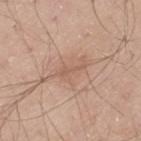Assessment: Recorded during total-body skin imaging; not selected for excision or biopsy. Image and clinical context: Captured under white-light illumination. A male subject, aged 58 to 62. The lesion is on the left thigh. A 15 mm close-up extracted from a 3D total-body photography capture.